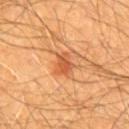Recorded during total-body skin imaging; not selected for excision or biopsy.
A male subject, in their 60s.
The lesion's longest dimension is about 3 mm.
A 15 mm crop from a total-body photograph taken for skin-cancer surveillance.
The tile uses cross-polarized illumination.
Automated tile analysis of the lesion measured a border-irregularity index near 3/10 and radial color variation of about 1. The software also gave a nevus-likeness score of about 95/100 and a lesion-detection confidence of about 100/100.
The lesion is on the chest.